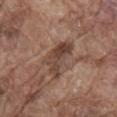Part of a total-body skin-imaging series; this lesion was reviewed on a skin check and was not flagged for biopsy. The lesion is located on the mid back. A male patient, aged 78–82. Captured under white-light illumination. Automated image analysis of the tile measured an average lesion color of about L≈42 a*≈18 b*≈25 (CIELAB), roughly 11 lightness units darker than nearby skin, and a normalized border contrast of about 8.5. The recorded lesion diameter is about 5 mm. A 15 mm close-up tile from a total-body photography series done for melanoma screening.